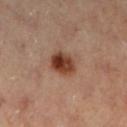Part of a total-body skin-imaging series; this lesion was reviewed on a skin check and was not flagged for biopsy.
On the right leg.
This image is a 15 mm lesion crop taken from a total-body photograph.
Captured under cross-polarized illumination.
A female patient about 60 years old.
Measured at roughly 3.5 mm in maximum diameter.